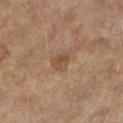{
  "biopsy_status": "not biopsied; imaged during a skin examination",
  "patient": {
    "sex": "female",
    "age_approx": 60
  },
  "automated_metrics": {
    "nevus_likeness_0_100": 5
  },
  "site": "leg",
  "image": {
    "source": "total-body photography crop",
    "field_of_view_mm": 15
  }
}